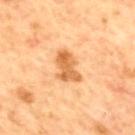Background:
Measured at roughly 4 mm in maximum diameter. A region of skin cropped from a whole-body photographic capture, roughly 15 mm wide. A male subject, about 60 years old. The lesion is located on the upper back. Imaged with cross-polarized lighting.